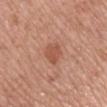<tbp_lesion>
  <biopsy_status>not biopsied; imaged during a skin examination</biopsy_status>
  <patient>
    <sex>male</sex>
    <age_approx>75</age_approx>
  </patient>
  <site>arm</site>
  <lesion_size>
    <long_diameter_mm_approx>2.5</long_diameter_mm_approx>
  </lesion_size>
  <automated_metrics>
    <cielab_L>53</cielab_L>
    <cielab_a>26</cielab_a>
    <cielab_b>31</cielab_b>
    <vs_skin_darker_L>8.0</vs_skin_darker_L>
    <border_irregularity_0_10>2.0</border_irregularity_0_10>
    <color_variation_0_10>2.0</color_variation_0_10>
    <peripheral_color_asymmetry>0.5</peripheral_color_asymmetry>
  </automated_metrics>
  <image>
    <source>total-body photography crop</source>
    <field_of_view_mm>15</field_of_view_mm>
  </image>
</tbp_lesion>